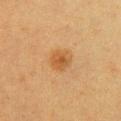<lesion>
  <biopsy_status>not biopsied; imaged during a skin examination</biopsy_status>
  <image>
    <source>total-body photography crop</source>
    <field_of_view_mm>15</field_of_view_mm>
  </image>
  <lesion_size>
    <long_diameter_mm_approx>2.5</long_diameter_mm_approx>
  </lesion_size>
  <patient>
    <sex>female</sex>
    <age_approx>40</age_approx>
  </patient>
  <site>chest</site>
  <lighting>cross-polarized</lighting>
</lesion>